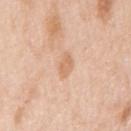No biopsy was performed on this lesion — it was imaged during a full skin examination and was not determined to be concerning.
Cropped from a total-body skin-imaging series; the visible field is about 15 mm.
The recorded lesion diameter is about 3 mm.
On the right upper arm.
A female patient, aged 43 to 47.
The lesion-visualizer software estimated a footprint of about 4 mm² and an outline eccentricity of about 0.75 (0 = round, 1 = elongated). The software also gave a lesion–skin lightness drop of about 8.
The tile uses white-light illumination.